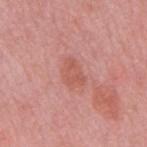Imaged with white-light lighting.
A 15 mm crop from a total-body photograph taken for skin-cancer surveillance.
A male patient, aged around 50.
Located on the mid back.
The recorded lesion diameter is about 3 mm.